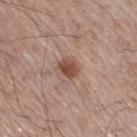{
  "biopsy_status": "not biopsied; imaged during a skin examination",
  "patient": {
    "sex": "male",
    "age_approx": 60
  },
  "lesion_size": {
    "long_diameter_mm_approx": 2.5
  },
  "automated_metrics": {
    "area_mm2_approx": 4.5,
    "eccentricity": 0.6,
    "shape_asymmetry": 0.15,
    "vs_skin_contrast_norm": 9.0,
    "nevus_likeness_0_100": 95,
    "lesion_detection_confidence_0_100": 100
  },
  "site": "right thigh",
  "lighting": "white-light",
  "image": {
    "source": "total-body photography crop",
    "field_of_view_mm": 15
  }
}